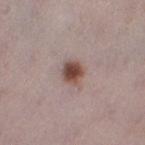{
  "patient": {
    "sex": "female",
    "age_approx": 30
  },
  "lesion_size": {
    "long_diameter_mm_approx": 2.5
  },
  "automated_metrics": {
    "vs_skin_darker_L": 15.0,
    "vs_skin_contrast_norm": 11.0,
    "border_irregularity_0_10": 1.5,
    "color_variation_0_10": 5.0,
    "peripheral_color_asymmetry": 1.5
  },
  "site": "left thigh",
  "image": {
    "source": "total-body photography crop",
    "field_of_view_mm": 15
  },
  "lighting": "white-light"
}